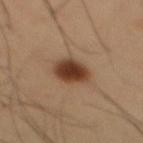The lesion was tiled from a total-body skin photograph and was not biopsied.
A male patient, in their mid-50s.
From the front of the torso.
This is a cross-polarized tile.
A roughly 15 mm field-of-view crop from a total-body skin photograph.
Measured at roughly 3.5 mm in maximum diameter.
The total-body-photography lesion software estimated an average lesion color of about L≈37 a*≈19 b*≈29 (CIELAB) and a normalized lesion–skin contrast near 12. It also reported a nevus-likeness score of about 100/100.